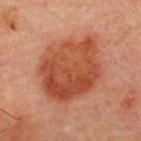Part of a total-body skin-imaging series; this lesion was reviewed on a skin check and was not flagged for biopsy. The subject is a male aged 58 to 62. The lesion is located on the upper back. Captured under cross-polarized illumination. A 15 mm close-up tile from a total-body photography series done for melanoma screening. Approximately 7.5 mm at its widest. Automated image analysis of the tile measured a lesion area of about 40 mm², an outline eccentricity of about 0.5 (0 = round, 1 = elongated), and a shape-asymmetry score of about 0.15 (0 = symmetric). And it measured a nevus-likeness score of about 80/100.